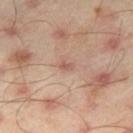This lesion was catalogued during total-body skin photography and was not selected for biopsy. Located on the right thigh. The subject is a male approximately 45 years of age. The tile uses cross-polarized illumination. The recorded lesion diameter is about 1.5 mm. A 15 mm crop from a total-body photograph taken for skin-cancer surveillance.